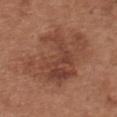Captured during whole-body skin photography for melanoma surveillance; the lesion was not biopsied. The patient is a female approximately 65 years of age. The lesion is on the chest. Imaged with white-light lighting. A 15 mm close-up extracted from a 3D total-body photography capture.